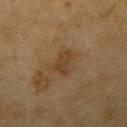The lesion was photographed on a routine skin check and not biopsied; there is no pathology result. A male patient, aged 58 to 62. The tile uses cross-polarized illumination. A 15 mm crop from a total-body photograph taken for skin-cancer surveillance. Measured at roughly 3 mm in maximum diameter. An algorithmic analysis of the crop reported a footprint of about 5 mm². The software also gave border irregularity of about 3.5 on a 0–10 scale. The lesion is located on the right upper arm.